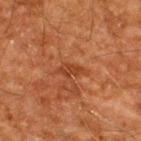workup — no biopsy performed (imaged during a skin exam)
lighting — cross-polarized illumination
image-analysis metrics — a lesion color around L≈32 a*≈23 b*≈31 in CIELAB; internal color variation of about 0 on a 0–10 scale and a peripheral color-asymmetry measure near 0; an automated nevus-likeness rating near 0 out of 100 and lesion-presence confidence of about 100/100
imaging modality — 15 mm crop, total-body photography
lesion diameter — about 2.5 mm
body site — the upper back
subject — male, aged approximately 60